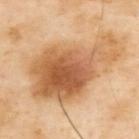<lesion>
  <biopsy_status>not biopsied; imaged during a skin examination</biopsy_status>
  <lesion_size>
    <long_diameter_mm_approx>10.5</long_diameter_mm_approx>
  </lesion_size>
  <patient>
    <sex>male</sex>
    <age_approx>55</age_approx>
  </patient>
  <image>
    <source>total-body photography crop</source>
    <field_of_view_mm>15</field_of_view_mm>
  </image>
  <site>back</site>
  <lighting>cross-polarized</lighting>
  <automated_metrics>
    <cielab_L>60</cielab_L>
    <cielab_a>22</cielab_a>
    <cielab_b>39</cielab_b>
    <vs_skin_darker_L>14.0</vs_skin_darker_L>
    <vs_skin_contrast_norm>9.0</vs_skin_contrast_norm>
    <border_irregularity_0_10>5.0</border_irregularity_0_10>
    <color_variation_0_10>8.5</color_variation_0_10>
    <nevus_likeness_0_100>90</nevus_likeness_0_100>
    <lesion_detection_confidence_0_100>100</lesion_detection_confidence_0_100>
  </automated_metrics>
</lesion>